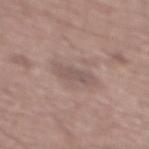– notes: total-body-photography surveillance lesion; no biopsy
– lesion size: ~4 mm (longest diameter)
– automated metrics: about 7 CIELAB-L* units darker than the surrounding skin and a lesion-to-skin contrast of about 5.5 (normalized; higher = more distinct); a color-variation rating of about 2/10 and radial color variation of about 1; a detector confidence of about 95 out of 100 that the crop contains a lesion
– patient: male, aged 58 to 62
– tile lighting: white-light illumination
– body site: the mid back
– imaging modality: ~15 mm crop, total-body skin-cancer survey